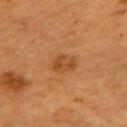site = the upper back
lesion size = about 3 mm
lighting = cross-polarized
imaging modality = 15 mm crop, total-body photography
patient = female, aged around 55